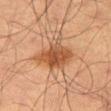{
  "biopsy_status": "not biopsied; imaged during a skin examination",
  "patient": {
    "sex": "male",
    "age_approx": 65
  },
  "image": {
    "source": "total-body photography crop",
    "field_of_view_mm": 15
  },
  "lesion_size": {
    "long_diameter_mm_approx": 5.5
  },
  "site": "left thigh",
  "automated_metrics": {
    "border_irregularity_0_10": 4.0,
    "color_variation_0_10": 4.0,
    "peripheral_color_asymmetry": 1.0
  },
  "lighting": "cross-polarized"
}